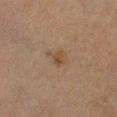This lesion was catalogued during total-body skin photography and was not selected for biopsy. Automated image analysis of the tile measured internal color variation of about 2.5 on a 0–10 scale and a peripheral color-asymmetry measure near 1. The analysis additionally found an automated nevus-likeness rating near 0 out of 100. The lesion is located on the left lower leg. A male subject aged around 65. A close-up tile cropped from a whole-body skin photograph, about 15 mm across.